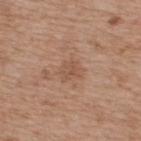Assessment: No biopsy was performed on this lesion — it was imaged during a full skin examination and was not determined to be concerning. Clinical summary: The lesion is located on the upper back. The patient is a male aged 63–67. Cropped from a total-body skin-imaging series; the visible field is about 15 mm. The total-body-photography lesion software estimated a mean CIELAB color near L≈53 a*≈20 b*≈30 and a lesion–skin lightness drop of about 7. The analysis additionally found a nevus-likeness score of about 0/100 and lesion-presence confidence of about 100/100.